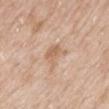No biopsy was performed on this lesion — it was imaged during a full skin examination and was not determined to be concerning. The lesion is on the mid back. The subject is a male aged around 65. The tile uses white-light illumination. A region of skin cropped from a whole-body photographic capture, roughly 15 mm wide. About 3 mm across.